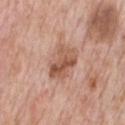{"biopsy_status": "not biopsied; imaged during a skin examination", "patient": {"sex": "male", "age_approx": 60}, "image": {"source": "total-body photography crop", "field_of_view_mm": 15}, "automated_metrics": {"area_mm2_approx": 10.0, "eccentricity": 0.75, "shape_asymmetry": 0.2, "cielab_L": 55, "cielab_a": 22, "cielab_b": 30, "vs_skin_darker_L": 11.0, "vs_skin_contrast_norm": 8.0, "border_irregularity_0_10": 2.5, "peripheral_color_asymmetry": 2.5}, "site": "chest", "lesion_size": {"long_diameter_mm_approx": 4.5}, "lighting": "white-light"}